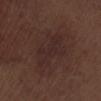workup: no biopsy performed (imaged during a skin exam); site: the left lower leg; tile lighting: white-light; subject: male, about 70 years old; image source: ~15 mm crop, total-body skin-cancer survey.